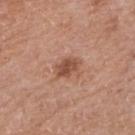Q: Was this lesion biopsied?
A: total-body-photography surveillance lesion; no biopsy
Q: How was this image acquired?
A: 15 mm crop, total-body photography
Q: Automated lesion metrics?
A: a footprint of about 5 mm², a shape eccentricity near 0.75, and two-axis asymmetry of about 0.2; border irregularity of about 2 on a 0–10 scale, internal color variation of about 2.5 on a 0–10 scale, and radial color variation of about 1
Q: What are the patient's age and sex?
A: female, in their 60s
Q: What is the lesion's diameter?
A: ~3.5 mm (longest diameter)
Q: What is the anatomic site?
A: the right upper arm